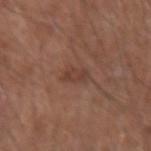Clinical impression: No biopsy was performed on this lesion — it was imaged during a full skin examination and was not determined to be concerning. Context: The patient is a male aged 63 to 67. Automated image analysis of the tile measured a lesion area of about 3 mm² and an eccentricity of roughly 0.9. And it measured a lesion color around L≈40 a*≈20 b*≈26 in CIELAB and a normalized lesion–skin contrast near 6. It also reported a color-variation rating of about 0.5/10 and radial color variation of about 0. The lesion's longest dimension is about 3 mm. A region of skin cropped from a whole-body photographic capture, roughly 15 mm wide. Captured under white-light illumination. From the right forearm.